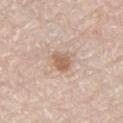Clinical summary: A close-up tile cropped from a whole-body skin photograph, about 15 mm across. Located on the left thigh. Imaged with white-light lighting. The subject is a male roughly 80 years of age.